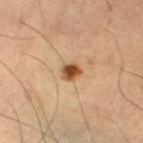The lesion was photographed on a routine skin check and not biopsied; there is no pathology result.
A lesion tile, about 15 mm wide, cut from a 3D total-body photograph.
The lesion is on the left lower leg.
The lesion-visualizer software estimated a mean CIELAB color near L≈50 a*≈22 b*≈36 and about 17 CIELAB-L* units darker than the surrounding skin. The analysis additionally found border irregularity of about 2 on a 0–10 scale and peripheral color asymmetry of about 1.5. And it measured a nevus-likeness score of about 100/100 and a lesion-detection confidence of about 100/100.
Longest diameter approximately 2.5 mm.
Captured under cross-polarized illumination.
A male subject in their mid-60s.